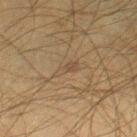notes = catalogued during a skin exam; not biopsied | patient = male, aged 48–52 | image = 15 mm crop, total-body photography | location = the right thigh | automated lesion analysis = roughly 5 lightness units darker than nearby skin and a normalized lesion–skin contrast near 4.5; a border-irregularity index near 4/10, internal color variation of about 1 on a 0–10 scale, and peripheral color asymmetry of about 0.5; a lesion-detection confidence of about 75/100 | tile lighting = cross-polarized | lesion diameter = ≈3 mm.